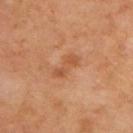Captured during whole-body skin photography for melanoma surveillance; the lesion was not biopsied. A male subject, aged 53 to 57. The recorded lesion diameter is about 4 mm. A roughly 15 mm field-of-view crop from a total-body skin photograph. Located on the back.